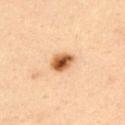Findings:
- follow-up: catalogued during a skin exam; not biopsied
- lighting: cross-polarized
- diameter: ≈3 mm
- location: the upper back
- patient: male, roughly 35 years of age
- imaging modality: ~15 mm tile from a whole-body skin photo
- automated lesion analysis: an automated nevus-likeness rating near 100 out of 100